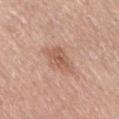| field | value |
|---|---|
| biopsy status | imaged on a skin check; not biopsied |